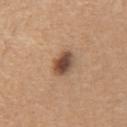This lesion was catalogued during total-body skin photography and was not selected for biopsy.
A female subject aged 58–62.
The tile uses white-light illumination.
A lesion tile, about 15 mm wide, cut from a 3D total-body photograph.
Measured at roughly 3 mm in maximum diameter.
The lesion is located on the arm.